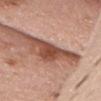notes = imaged on a skin check; not biopsied | location = the head or neck | image source = 15 mm crop, total-body photography | tile lighting = white-light | lesion size = ~7 mm (longest diameter) | image-analysis metrics = a border-irregularity index near 7.5/10 and peripheral color asymmetry of about 0.5 | patient = female, aged 58–62.